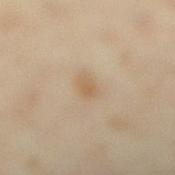workup: catalogued during a skin exam; not biopsied | diameter: about 2.5 mm | acquisition: ~15 mm tile from a whole-body skin photo | patient: female, aged 33 to 37 | lighting: cross-polarized | location: the right lower leg | automated metrics: a lesion area of about 3 mm² and an outline eccentricity of about 0.8 (0 = round, 1 = elongated).